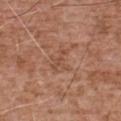Findings:
– follow-up · catalogued during a skin exam; not biopsied
– acquisition · 15 mm crop, total-body photography
– subject · male, aged 73 to 77
– illumination · white-light illumination
– anatomic site · the chest
– TBP lesion metrics · peripheral color asymmetry of about 0; a classifier nevus-likeness of about 0/100 and a detector confidence of about 100 out of 100 that the crop contains a lesion
– lesion diameter · ≈3 mm